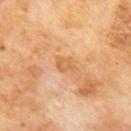Clinical impression: Imaged during a routine full-body skin examination; the lesion was not biopsied and no histopathology is available. Image and clinical context: The lesion-visualizer software estimated a lesion area of about 3.5 mm², an eccentricity of roughly 0.75, and a shape-asymmetry score of about 0.35 (0 = symmetric). And it measured a lesion color around L≈62 a*≈23 b*≈41 in CIELAB and a normalized border contrast of about 5.5. And it measured a border-irregularity rating of about 3/10, a within-lesion color-variation index near 2/10, and radial color variation of about 0.5. Imaged with cross-polarized lighting. This image is a 15 mm lesion crop taken from a total-body photograph. On the mid back. The patient is a male in their 70s.